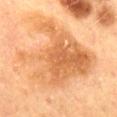<record>
  <biopsy_status>not biopsied; imaged during a skin examination</biopsy_status>
  <lesion_size>
    <long_diameter_mm_approx>9.0</long_diameter_mm_approx>
  </lesion_size>
  <site>back</site>
  <image>
    <source>total-body photography crop</source>
    <field_of_view_mm>15</field_of_view_mm>
  </image>
  <automated_metrics>
    <nevus_likeness_0_100>25</nevus_likeness_0_100>
    <lesion_detection_confidence_0_100>100</lesion_detection_confidence_0_100>
  </automated_metrics>
  <patient>
    <sex>female</sex>
    <age_approx>55</age_approx>
  </patient>
  <lighting>cross-polarized</lighting>
</record>